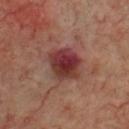Part of a total-body skin-imaging series; this lesion was reviewed on a skin check and was not flagged for biopsy.
The tile uses cross-polarized illumination.
Longest diameter approximately 4 mm.
A male patient in their 70s.
From the front of the torso.
A close-up tile cropped from a whole-body skin photograph, about 15 mm across.